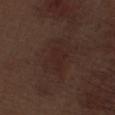patient: male, aged around 70 | location: the right thigh | tile lighting: white-light | lesion size: ~5 mm (longest diameter) | image source: ~15 mm tile from a whole-body skin photo.